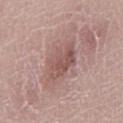No biopsy was performed on this lesion — it was imaged during a full skin examination and was not determined to be concerning. The tile uses white-light illumination. An algorithmic analysis of the crop reported a footprint of about 11 mm², an outline eccentricity of about 0.75 (0 = round, 1 = elongated), and a symmetry-axis asymmetry near 0.25. The software also gave a normalized lesion–skin contrast near 6.5. It also reported border irregularity of about 4 on a 0–10 scale, a within-lesion color-variation index near 3.5/10, and a peripheral color-asymmetry measure near 1.5. It also reported lesion-presence confidence of about 90/100. A female patient roughly 30 years of age. A close-up tile cropped from a whole-body skin photograph, about 15 mm across. The lesion is located on the right lower leg. Longest diameter approximately 4.5 mm.